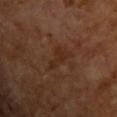{"site": "chest", "lighting": "cross-polarized", "patient": {"sex": "male", "age_approx": 65}, "image": {"source": "total-body photography crop", "field_of_view_mm": 15}, "lesion_size": {"long_diameter_mm_approx": 4.5}}